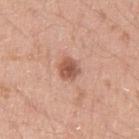notes=total-body-photography surveillance lesion; no biopsy | acquisition=~15 mm crop, total-body skin-cancer survey | illumination=white-light | anatomic site=the left upper arm | patient=male, aged around 20.